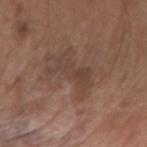This is a white-light tile. The patient is a male aged 68–72. Automated tile analysis of the lesion measured a shape eccentricity near 0.95. It also reported a border-irregularity index near 7.5/10, internal color variation of about 1 on a 0–10 scale, and a peripheral color-asymmetry measure near 0. Longest diameter approximately 5.5 mm. The lesion is located on the right forearm. A region of skin cropped from a whole-body photographic capture, roughly 15 mm wide.